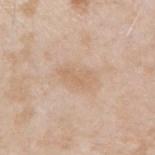Assessment:
The lesion was tiled from a total-body skin photograph and was not biopsied.
Acquisition and patient details:
Imaged with white-light lighting. The lesion is located on the right upper arm. A male patient, about 45 years old. Approximately 4 mm at its widest. A 15 mm close-up extracted from a 3D total-body photography capture.